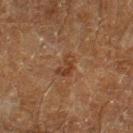| key | value |
|---|---|
| biopsy status | imaged on a skin check; not biopsied |
| patient | male, aged around 60 |
| lighting | cross-polarized illumination |
| site | the right lower leg |
| imaging modality | ~15 mm tile from a whole-body skin photo |
| lesion diameter | ≈3 mm |